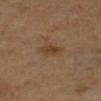Notes:
• size — ~3 mm (longest diameter)
• lighting — cross-polarized illumination
• subject — female, aged approximately 30
• TBP lesion metrics — a lesion area of about 4 mm²; a border-irregularity index near 2/10, a within-lesion color-variation index near 2/10, and radial color variation of about 1
• image — ~15 mm crop, total-body skin-cancer survey
• anatomic site — the right lower leg